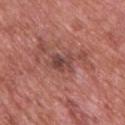notes — imaged on a skin check; not biopsied
image source — total-body-photography crop, ~15 mm field of view
anatomic site — the upper back
lesion diameter — ~3 mm (longest diameter)
subject — male, aged 68–72
image-analysis metrics — a border-irregularity rating of about 3/10, a color-variation rating of about 4/10, and a peripheral color-asymmetry measure near 1.5; a classifier nevus-likeness of about 0/100 and lesion-presence confidence of about 100/100
tile lighting — white-light illumination